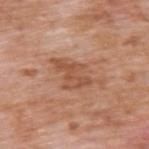Notes:
* notes · imaged on a skin check; not biopsied
* site · the upper back
* lesion diameter · ≈6 mm
* lighting · white-light
* patient · male, in their 60s
* image-analysis metrics · about 9 CIELAB-L* units darker than the surrounding skin
* image source · 15 mm crop, total-body photography